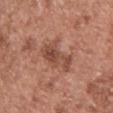Clinical summary:
The lesion is on the chest. Approximately 5.5 mm at its widest. The tile uses white-light illumination. The patient is a male approximately 70 years of age. A 15 mm close-up tile from a total-body photography series done for melanoma screening.